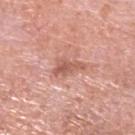notes: no biopsy performed (imaged during a skin exam)
lesion size: ~4 mm (longest diameter)
body site: the head or neck
imaging modality: ~15 mm tile from a whole-body skin photo
patient: male, approximately 80 years of age
lighting: white-light illumination